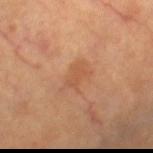| field | value |
|---|---|
| body site | the leg |
| imaging modality | total-body-photography crop, ~15 mm field of view |
| TBP lesion metrics | a classifier nevus-likeness of about 10/100 and a detector confidence of about 100 out of 100 that the crop contains a lesion |
| patient | female, aged around 70 |
| lighting | cross-polarized illumination |
| size | ≈2.5 mm |